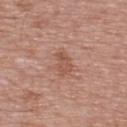Recorded during total-body skin imaging; not selected for excision or biopsy.
Imaged with white-light lighting.
Cropped from a whole-body photographic skin survey; the tile spans about 15 mm.
On the upper back.
A male subject aged 63 to 67.